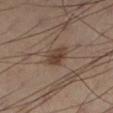follow-up = total-body-photography surveillance lesion; no biopsy